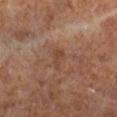Imaged during a routine full-body skin examination; the lesion was not biopsied and no histopathology is available.
Measured at roughly 2.5 mm in maximum diameter.
A male patient aged 58–62.
Automated tile analysis of the lesion measured a footprint of about 3.5 mm² and a shape eccentricity near 0.85. And it measured an average lesion color of about L≈42 a*≈19 b*≈29 (CIELAB), about 5 CIELAB-L* units darker than the surrounding skin, and a lesion-to-skin contrast of about 5 (normalized; higher = more distinct). The software also gave lesion-presence confidence of about 100/100.
A 15 mm crop from a total-body photograph taken for skin-cancer surveillance.
Captured under cross-polarized illumination.
From the right lower leg.